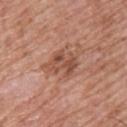Image and clinical context:
A male subject, aged around 65. Located on the upper back. A region of skin cropped from a whole-body photographic capture, roughly 15 mm wide.